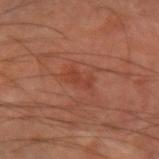notes: no biopsy performed (imaged during a skin exam)
image: ~15 mm crop, total-body skin-cancer survey
patient: male, roughly 70 years of age
size: ~3 mm (longest diameter)
anatomic site: the right forearm
TBP lesion metrics: an outline eccentricity of about 0.9 (0 = round, 1 = elongated) and a symmetry-axis asymmetry near 0.45; a mean CIELAB color near L≈30 a*≈22 b*≈25; a border-irregularity index near 5/10 and peripheral color asymmetry of about 0; a classifier nevus-likeness of about 0/100 and lesion-presence confidence of about 100/100